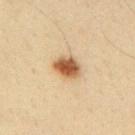notes: total-body-photography surveillance lesion; no biopsy | location: the upper back | patient: male, aged 33–37 | image: ~15 mm crop, total-body skin-cancer survey.